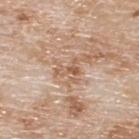Assessment:
Imaged during a routine full-body skin examination; the lesion was not biopsied and no histopathology is available.
Image and clinical context:
From the back. A male subject, aged around 80. A 15 mm crop from a total-body photograph taken for skin-cancer surveillance.